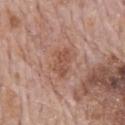The lesion was tiled from a total-body skin photograph and was not biopsied.
Automated tile analysis of the lesion measured a lesion area of about 6 mm², an eccentricity of roughly 0.8, and two-axis asymmetry of about 0.25. The software also gave a lesion color around L≈51 a*≈22 b*≈28 in CIELAB and a normalized lesion–skin contrast near 6. And it measured a border-irregularity index near 2.5/10, a color-variation rating of about 2.5/10, and peripheral color asymmetry of about 1.
Longest diameter approximately 3.5 mm.
A 15 mm close-up tile from a total-body photography series done for melanoma screening.
Located on the mid back.
A male patient, approximately 70 years of age.
This is a white-light tile.